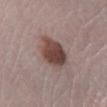Context: A 15 mm close-up tile from a total-body photography series done for melanoma screening. The lesion is on the left lower leg. A female subject, roughly 40 years of age. The total-body-photography lesion software estimated a footprint of about 13 mm² and an eccentricity of roughly 0.6. And it measured a border-irregularity index near 2/10, a within-lesion color-variation index near 4.5/10, and radial color variation of about 1.5. It also reported an automated nevus-likeness rating near 95 out of 100.